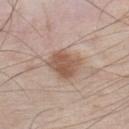follow-up — no biopsy performed (imaged during a skin exam)
imaging modality — ~15 mm crop, total-body skin-cancer survey
tile lighting — white-light
lesion diameter — ≈3.5 mm
subject — male, aged 58–62
site — the left lower leg
automated metrics — a lesion area of about 10 mm² and an eccentricity of roughly 0.5; a mean CIELAB color near L≈55 a*≈18 b*≈28 and roughly 11 lightness units darker than nearby skin; a border-irregularity rating of about 2/10, internal color variation of about 3 on a 0–10 scale, and peripheral color asymmetry of about 1